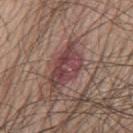workup = total-body-photography surveillance lesion; no biopsy.